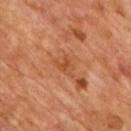Assessment:
Imaged during a routine full-body skin examination; the lesion was not biopsied and no histopathology is available.
Context:
A male patient aged approximately 65. A lesion tile, about 15 mm wide, cut from a 3D total-body photograph.